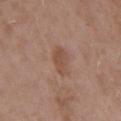  biopsy_status: not biopsied; imaged during a skin examination
  patient:
    sex: male
    age_approx: 55
  site: arm
  lesion_size:
    long_diameter_mm_approx: 3.5
  image:
    source: total-body photography crop
    field_of_view_mm: 15